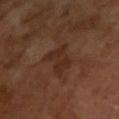| key | value |
|---|---|
| follow-up | no biopsy performed (imaged during a skin exam) |
| patient | male, in their mid- to late 60s |
| lighting | cross-polarized illumination |
| image | total-body-photography crop, ~15 mm field of view |
| lesion diameter | ~5 mm (longest diameter) |